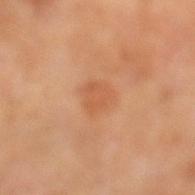Clinical impression: Imaged during a routine full-body skin examination; the lesion was not biopsied and no histopathology is available. Background: The subject is a male roughly 65 years of age. Located on the left lower leg. This image is a 15 mm lesion crop taken from a total-body photograph.